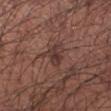biopsy status: total-body-photography surveillance lesion; no biopsy
body site: the left forearm
patient: male, approximately 55 years of age
image source: 15 mm crop, total-body photography
lighting: white-light illumination
diameter: ≈3 mm